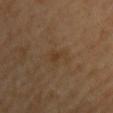{
  "lighting": "cross-polarized",
  "image": {
    "source": "total-body photography crop",
    "field_of_view_mm": 15
  },
  "patient": {
    "sex": "male",
    "age_approx": 40
  },
  "automated_metrics": {
    "cielab_L": 37,
    "cielab_a": 16,
    "cielab_b": 30,
    "vs_skin_darker_L": 5.0,
    "vs_skin_contrast_norm": 5.5,
    "nevus_likeness_0_100": 0
  },
  "site": "chest",
  "lesion_size": {
    "long_diameter_mm_approx": 2.5
  }
}